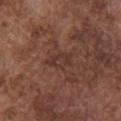Imaged during a routine full-body skin examination; the lesion was not biopsied and no histopathology is available. Automated tile analysis of the lesion measured a lesion color around L≈34 a*≈20 b*≈22 in CIELAB, about 6 CIELAB-L* units darker than the surrounding skin, and a normalized border contrast of about 6. The analysis additionally found a border-irregularity index near 3.5/10, a color-variation rating of about 2.5/10, and a peripheral color-asymmetry measure near 1. And it measured lesion-presence confidence of about 100/100. The lesion's longest dimension is about 3 mm. A lesion tile, about 15 mm wide, cut from a 3D total-body photograph. From the chest. The subject is a male aged around 75.